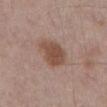Q: Was a biopsy performed?
A: catalogued during a skin exam; not biopsied
Q: Lesion location?
A: the abdomen
Q: What kind of image is this?
A: total-body-photography crop, ~15 mm field of view
Q: Illumination type?
A: white-light
Q: Lesion size?
A: ~3.5 mm (longest diameter)
Q: What are the patient's age and sex?
A: male, approximately 55 years of age
Q: Automated lesion metrics?
A: an area of roughly 9.5 mm², a shape eccentricity near 0.5, and a symmetry-axis asymmetry near 0.25; a lesion color around L≈48 a*≈19 b*≈27 in CIELAB and a normalized border contrast of about 8.5; an automated nevus-likeness rating near 95 out of 100 and a detector confidence of about 100 out of 100 that the crop contains a lesion